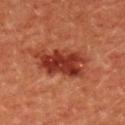biopsy status: total-body-photography surveillance lesion; no biopsy | automated metrics: a footprint of about 17 mm², a shape eccentricity near 0.85, and a shape-asymmetry score of about 0.25 (0 = symmetric); a lesion color around L≈34 a*≈30 b*≈30 in CIELAB, roughly 12 lightness units darker than nearby skin, and a normalized lesion–skin contrast near 10; a border-irregularity index near 3.5/10, a within-lesion color-variation index near 5/10, and radial color variation of about 2 | image source: ~15 mm crop, total-body skin-cancer survey | tile lighting: cross-polarized illumination | location: the left upper arm | subject: male, aged 63 to 67 | size: ~6 mm (longest diameter).